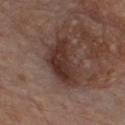This lesion was catalogued during total-body skin photography and was not selected for biopsy.
About 6 mm across.
A lesion tile, about 15 mm wide, cut from a 3D total-body photograph.
From the chest.
A male subject in their 80s.
Imaged with white-light lighting.